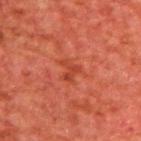Captured during whole-body skin photography for melanoma surveillance; the lesion was not biopsied.